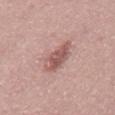Q: Was this lesion biopsied?
A: total-body-photography surveillance lesion; no biopsy
Q: How was the tile lit?
A: white-light
Q: Lesion size?
A: about 5 mm
Q: What are the patient's age and sex?
A: male, about 60 years old
Q: What is the anatomic site?
A: the front of the torso
Q: How was this image acquired?
A: total-body-photography crop, ~15 mm field of view